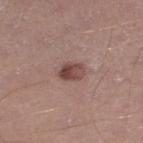patient = male, aged 38–42 | imaging modality = total-body-photography crop, ~15 mm field of view | lesion size = about 3 mm | tile lighting = white-light illumination | site = the leg.